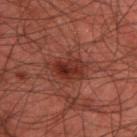Findings:
• follow-up — imaged on a skin check; not biopsied
• site — the back
• subject — male, roughly 45 years of age
• lesion size — ~4.5 mm (longest diameter)
• imaging modality — total-body-photography crop, ~15 mm field of view
• illumination — cross-polarized illumination